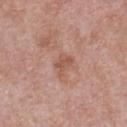No biopsy was performed on this lesion — it was imaged during a full skin examination and was not determined to be concerning. The lesion is located on the chest. Longest diameter approximately 3 mm. This is a white-light tile. Cropped from a whole-body photographic skin survey; the tile spans about 15 mm. An algorithmic analysis of the crop reported an area of roughly 4 mm², an eccentricity of roughly 0.75, and a symmetry-axis asymmetry near 0.4. A male subject, in their mid- to late 50s.